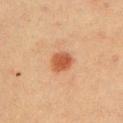Imaged during a routine full-body skin examination; the lesion was not biopsied and no histopathology is available. A male patient, about 40 years old. The lesion is located on the left upper arm. Imaged with cross-polarized lighting. Measured at roughly 3 mm in maximum diameter. Cropped from a total-body skin-imaging series; the visible field is about 15 mm.